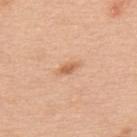| key | value |
|---|---|
| workup | imaged on a skin check; not biopsied |
| image | ~15 mm crop, total-body skin-cancer survey |
| body site | the upper back |
| tile lighting | white-light |
| subject | male, in their 50s |
| diameter | ≈2.5 mm |